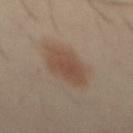The lesion is on the abdomen.
The tile uses cross-polarized illumination.
Cropped from a whole-body photographic skin survey; the tile spans about 15 mm.
The lesion-visualizer software estimated a footprint of about 16 mm², an eccentricity of roughly 0.85, and a symmetry-axis asymmetry near 0.1. It also reported an automated nevus-likeness rating near 100 out of 100 and lesion-presence confidence of about 100/100.
A male patient, roughly 50 years of age.